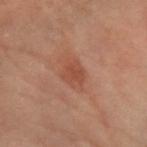Clinical impression:
Imaged during a routine full-body skin examination; the lesion was not biopsied and no histopathology is available.
Background:
About 3.5 mm across. Cropped from a total-body skin-imaging series; the visible field is about 15 mm. The subject is a female aged 58 to 62. Located on the arm. This is a cross-polarized tile.